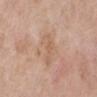imaging modality: 15 mm crop, total-body photography
subject: female, about 70 years old
site: the left lower leg
tile lighting: white-light illumination
lesion size: about 4 mm